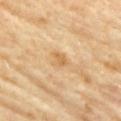Image and clinical context:
An algorithmic analysis of the crop reported a lesion area of about 3.5 mm² and an eccentricity of roughly 0.7. This is a cross-polarized tile. On the front of the torso. A region of skin cropped from a whole-body photographic capture, roughly 15 mm wide. A female subject, aged 73 to 77.